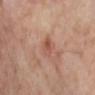Impression: Captured during whole-body skin photography for melanoma surveillance; the lesion was not biopsied. Context: Cropped from a whole-body photographic skin survey; the tile spans about 15 mm. Approximately 2.5 mm at its widest. The tile uses cross-polarized illumination. On the left lower leg. Automated image analysis of the tile measured a mean CIELAB color near L≈54 a*≈24 b*≈29, a lesion–skin lightness drop of about 8, and a normalized border contrast of about 6. The analysis additionally found an automated nevus-likeness rating near 5 out of 100 and a detector confidence of about 100 out of 100 that the crop contains a lesion. A female subject, about 55 years old.